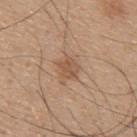follow-up: no biopsy performed (imaged during a skin exam) | anatomic site: the upper back | imaging modality: 15 mm crop, total-body photography | patient: male, aged 43 to 47.